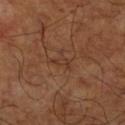Impression: Captured during whole-body skin photography for melanoma surveillance; the lesion was not biopsied. Context: The recorded lesion diameter is about 2.5 mm. This is a cross-polarized tile. A male patient aged 58–62. Automated tile analysis of the lesion measured an outline eccentricity of about 0.9 (0 = round, 1 = elongated) and a symmetry-axis asymmetry near 0.65. Cropped from a whole-body photographic skin survey; the tile spans about 15 mm. On the right lower leg.